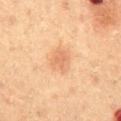<tbp_lesion>
<biopsy_status>not biopsied; imaged during a skin examination</biopsy_status>
<lighting>cross-polarized</lighting>
<image>
  <source>total-body photography crop</source>
  <field_of_view_mm>15</field_of_view_mm>
</image>
<automated_metrics>
  <color_variation_0_10>2.0</color_variation_0_10>
  <peripheral_color_asymmetry>0.5</peripheral_color_asymmetry>
</automated_metrics>
<patient>
  <sex>male</sex>
  <age_approx>60</age_approx>
</patient>
<lesion_size>
  <long_diameter_mm_approx>3.0</long_diameter_mm_approx>
</lesion_size>
<site>abdomen</site>
</tbp_lesion>